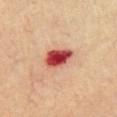Recorded during total-body skin imaging; not selected for excision or biopsy. Automated image analysis of the tile measured border irregularity of about 1.5 on a 0–10 scale, internal color variation of about 6 on a 0–10 scale, and a peripheral color-asymmetry measure near 1.5. The analysis additionally found a nevus-likeness score of about 0/100 and a lesion-detection confidence of about 100/100. The lesion is on the chest. This is a cross-polarized tile. This image is a 15 mm lesion crop taken from a total-body photograph. The lesion's longest dimension is about 4 mm. A male subject aged around 70.